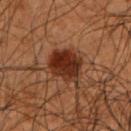| field | value |
|---|---|
| biopsy status | catalogued during a skin exam; not biopsied |
| illumination | cross-polarized |
| body site | the left upper arm |
| imaging modality | ~15 mm crop, total-body skin-cancer survey |
| subject | male, in their 50s |
| image-analysis metrics | a footprint of about 12 mm², an outline eccentricity of about 0.6 (0 = round, 1 = elongated), and two-axis asymmetry of about 0.15; a classifier nevus-likeness of about 95/100 and a detector confidence of about 100 out of 100 that the crop contains a lesion |